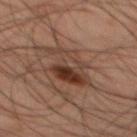Part of a total-body skin-imaging series; this lesion was reviewed on a skin check and was not flagged for biopsy. On the mid back. The lesion-visualizer software estimated an area of roughly 13 mm², an eccentricity of roughly 0.8, and a symmetry-axis asymmetry near 0.35. It also reported border irregularity of about 5 on a 0–10 scale and peripheral color asymmetry of about 4. It also reported a classifier nevus-likeness of about 85/100 and a detector confidence of about 100 out of 100 that the crop contains a lesion. A male subject aged around 45. A roughly 15 mm field-of-view crop from a total-body skin photograph. Captured under cross-polarized illumination. Approximately 6 mm at its widest.